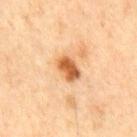Q: Is there a histopathology result?
A: imaged on a skin check; not biopsied
Q: What is the lesion's diameter?
A: ~3 mm (longest diameter)
Q: Where on the body is the lesion?
A: the front of the torso
Q: Who is the patient?
A: male, aged around 65
Q: How was this image acquired?
A: ~15 mm tile from a whole-body skin photo
Q: How was the tile lit?
A: cross-polarized illumination